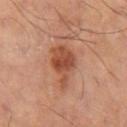The tile uses cross-polarized illumination.
About 5.5 mm across.
A close-up tile cropped from a whole-body skin photograph, about 15 mm across.
From the left thigh.
The lesion-visualizer software estimated a border-irregularity index near 4.5/10 and a color-variation rating of about 4/10.